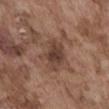This lesion was catalogued during total-body skin photography and was not selected for biopsy. A male subject aged 73–77. A region of skin cropped from a whole-body photographic capture, roughly 15 mm wide. The total-body-photography lesion software estimated a lesion area of about 9 mm², a shape eccentricity near 0.8, and two-axis asymmetry of about 0.25. It also reported border irregularity of about 3 on a 0–10 scale, internal color variation of about 3 on a 0–10 scale, and a peripheral color-asymmetry measure near 1. The analysis additionally found a classifier nevus-likeness of about 35/100 and lesion-presence confidence of about 100/100. This is a white-light tile. The lesion is located on the abdomen. Measured at roughly 4.5 mm in maximum diameter.